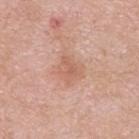Clinical summary: The recorded lesion diameter is about 3 mm. The patient is a male in their 60s. Captured under white-light illumination. The total-body-photography lesion software estimated a lesion area of about 6 mm² and an outline eccentricity of about 0.55 (0 = round, 1 = elongated). And it measured an average lesion color of about L≈61 a*≈23 b*≈30 (CIELAB), about 7 CIELAB-L* units darker than the surrounding skin, and a normalized lesion–skin contrast near 5.5. And it measured border irregularity of about 3 on a 0–10 scale and internal color variation of about 2.5 on a 0–10 scale. It also reported a lesion-detection confidence of about 100/100. The lesion is located on the upper back. A lesion tile, about 15 mm wide, cut from a 3D total-body photograph.